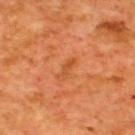{"biopsy_status": "not biopsied; imaged during a skin examination", "lighting": "cross-polarized", "image": {"source": "total-body photography crop", "field_of_view_mm": 15}, "site": "upper back", "patient": {"sex": "male", "age_approx": 65}}